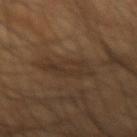Case summary:
– notes · total-body-photography surveillance lesion; no biopsy
– tile lighting · cross-polarized
– TBP lesion metrics · an average lesion color of about L≈32 a*≈14 b*≈26 (CIELAB); border irregularity of about 4 on a 0–10 scale, internal color variation of about 3 on a 0–10 scale, and a peripheral color-asymmetry measure near 1; a lesion-detection confidence of about 60/100
– image · ~15 mm crop, total-body skin-cancer survey
– site · the right forearm
– lesion size · about 5.5 mm
– subject · male, roughly 65 years of age